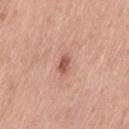The lesion was photographed on a routine skin check and not biopsied; there is no pathology result.
Cropped from a total-body skin-imaging series; the visible field is about 15 mm.
An algorithmic analysis of the crop reported a lesion area of about 3 mm², an eccentricity of roughly 0.8, and a shape-asymmetry score of about 0.25 (0 = symmetric). The analysis additionally found a border-irregularity index near 2/10, a color-variation rating of about 2/10, and a peripheral color-asymmetry measure near 1. It also reported a detector confidence of about 100 out of 100 that the crop contains a lesion.
From the left thigh.
The patient is a female in their mid-50s.
Approximately 2.5 mm at its widest.